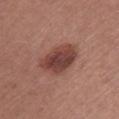  biopsy_status: not biopsied; imaged during a skin examination
  lesion_size:
    long_diameter_mm_approx: 5.0
  automated_metrics:
    cielab_L: 42
    cielab_a: 23
    cielab_b: 24
    vs_skin_darker_L: 13.0
    vs_skin_contrast_norm: 9.5
    nevus_likeness_0_100: 85
    lesion_detection_confidence_0_100: 100
  image:
    source: total-body photography crop
    field_of_view_mm: 15
  site: front of the torso
  patient:
    sex: female
    age_approx: 25
  lighting: white-light